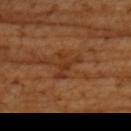The lesion was photographed on a routine skin check and not biopsied; there is no pathology result. The lesion is located on the upper back. A female patient about 55 years old. A 15 mm crop from a total-body photograph taken for skin-cancer surveillance. Automated tile analysis of the lesion measured a border-irregularity rating of about 9/10 and peripheral color asymmetry of about 0. The software also gave a classifier nevus-likeness of about 0/100 and a detector confidence of about 100 out of 100 that the crop contains a lesion. The tile uses cross-polarized illumination.